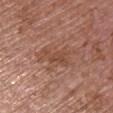<tbp_lesion>
  <biopsy_status>not biopsied; imaged during a skin examination</biopsy_status>
  <lesion_size>
    <long_diameter_mm_approx>3.5</long_diameter_mm_approx>
  </lesion_size>
  <patient>
    <sex>female</sex>
    <age_approx>65</age_approx>
  </patient>
  <site>chest</site>
  <image>
    <source>total-body photography crop</source>
    <field_of_view_mm>15</field_of_view_mm>
  </image>
</tbp_lesion>